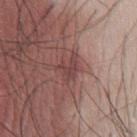follow-up: imaged on a skin check; not biopsied
TBP lesion metrics: an average lesion color of about L≈43 a*≈22 b*≈20 (CIELAB), about 8 CIELAB-L* units darker than the surrounding skin, and a normalized border contrast of about 6.5
acquisition: 15 mm crop, total-body photography
subject: male, aged approximately 50
illumination: white-light illumination
anatomic site: the chest